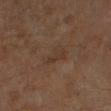No biopsy was performed on this lesion — it was imaged during a full skin examination and was not determined to be concerning. Longest diameter approximately 3.5 mm. Captured under cross-polarized illumination. A lesion tile, about 15 mm wide, cut from a 3D total-body photograph. The lesion is located on the leg. The subject is a male in their 60s.